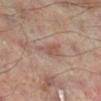This lesion was catalogued during total-body skin photography and was not selected for biopsy.
A region of skin cropped from a whole-body photographic capture, roughly 15 mm wide.
Captured under cross-polarized illumination.
Approximately 3.5 mm at its widest.
From the right lower leg.
A male patient, in their mid- to late 60s.